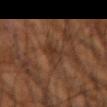Case summary:
– workup — no biopsy performed (imaged during a skin exam)
– lighting — cross-polarized
– imaging modality — ~15 mm tile from a whole-body skin photo
– subject — male, in their mid-60s
– automated lesion analysis — an automated nevus-likeness rating near 0 out of 100 and a lesion-detection confidence of about 60/100
– anatomic site — the arm
– diameter — ≈3.5 mm